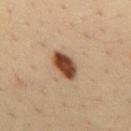Captured during whole-body skin photography for melanoma surveillance; the lesion was not biopsied.
Captured under cross-polarized illumination.
A 15 mm close-up tile from a total-body photography series done for melanoma screening.
From the mid back.
A male patient, about 35 years old.
Measured at roughly 4 mm in maximum diameter.